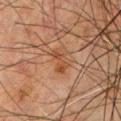Imaged during a routine full-body skin examination; the lesion was not biopsied and no histopathology is available.
Imaged with cross-polarized lighting.
This image is a 15 mm lesion crop taken from a total-body photograph.
Approximately 3 mm at its widest.
The patient is a male in their mid-60s.
Located on the chest.